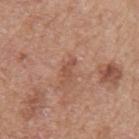Assessment:
No biopsy was performed on this lesion — it was imaged during a full skin examination and was not determined to be concerning.
Image and clinical context:
The lesion-visualizer software estimated a lesion area of about 2.5 mm², a shape eccentricity near 0.9, and a shape-asymmetry score of about 0.45 (0 = symmetric). The analysis additionally found a nevus-likeness score of about 0/100 and a detector confidence of about 100 out of 100 that the crop contains a lesion. A female patient, aged 53 to 57. Approximately 2.5 mm at its widest. This image is a 15 mm lesion crop taken from a total-body photograph. The lesion is on the arm.